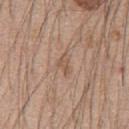follow-up = catalogued during a skin exam; not biopsied